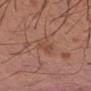Q: Was this lesion biopsied?
A: catalogued during a skin exam; not biopsied
Q: Lesion location?
A: the left forearm
Q: What is the lesion's diameter?
A: ~3.5 mm (longest diameter)
Q: What is the imaging modality?
A: ~15 mm tile from a whole-body skin photo
Q: What lighting was used for the tile?
A: white-light
Q: Who is the patient?
A: male, aged 28–32